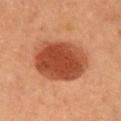notes — imaged on a skin check; not biopsied
diameter — about 7 mm
subject — female
tile lighting — cross-polarized illumination
site — the chest
imaging modality — ~15 mm crop, total-body skin-cancer survey
automated metrics — a footprint of about 29 mm², a shape eccentricity near 0.7, and two-axis asymmetry of about 0.1; a lesion color around L≈49 a*≈31 b*≈38 in CIELAB, roughly 16 lightness units darker than nearby skin, and a normalized border contrast of about 10.5; border irregularity of about 1 on a 0–10 scale, internal color variation of about 4.5 on a 0–10 scale, and radial color variation of about 1.5